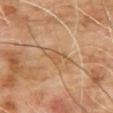| feature | finding |
|---|---|
| location | the upper back |
| patient | male, aged around 65 |
| image source | ~15 mm crop, total-body skin-cancer survey |
| automated metrics | a symmetry-axis asymmetry near 0.45 |
| lesion size | ~4 mm (longest diameter) |